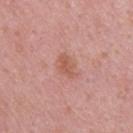Notes:
– follow-up · no biopsy performed (imaged during a skin exam)
– lighting · white-light
– image source · total-body-photography crop, ~15 mm field of view
– location · the upper back
– subject · male, in their 50s
– lesion diameter · ≈3 mm